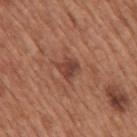Clinical impression:
The lesion was tiled from a total-body skin photograph and was not biopsied.
Background:
A 15 mm close-up tile from a total-body photography series done for melanoma screening. The subject is a male aged around 65. The lesion is located on the mid back.